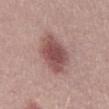Q: Was a biopsy performed?
A: imaged on a skin check; not biopsied
Q: What did automated image analysis measure?
A: a shape eccentricity near 0.85 and two-axis asymmetry of about 0.2; a color-variation rating of about 4/10 and radial color variation of about 1; a nevus-likeness score of about 85/100 and a lesion-detection confidence of about 100/100
Q: Patient demographics?
A: male, about 30 years old
Q: Lesion size?
A: ≈6.5 mm
Q: Illumination type?
A: white-light illumination
Q: What kind of image is this?
A: ~15 mm crop, total-body skin-cancer survey